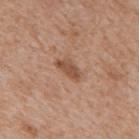Q: Is there a histopathology result?
A: total-body-photography surveillance lesion; no biopsy
Q: Illumination type?
A: white-light illumination
Q: What is the anatomic site?
A: the mid back
Q: Patient demographics?
A: male, approximately 65 years of age
Q: What is the lesion's diameter?
A: about 3.5 mm
Q: What did automated image analysis measure?
A: a nevus-likeness score of about 70/100 and a detector confidence of about 100 out of 100 that the crop contains a lesion
Q: What is the imaging modality?
A: 15 mm crop, total-body photography